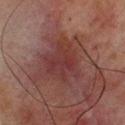biopsy status = catalogued during a skin exam; not biopsied
lighting = cross-polarized
subject = male, roughly 65 years of age
imaging modality = total-body-photography crop, ~15 mm field of view
body site = the upper back
lesion diameter = ~5.5 mm (longest diameter)